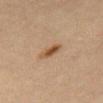No biopsy was performed on this lesion — it was imaged during a full skin examination and was not determined to be concerning. The tile uses cross-polarized illumination. On the abdomen. Automated image analysis of the tile measured a footprint of about 3.5 mm², an eccentricity of roughly 0.9, and a symmetry-axis asymmetry near 0.25. It also reported an automated nevus-likeness rating near 95 out of 100 and a lesion-detection confidence of about 100/100. A close-up tile cropped from a whole-body skin photograph, about 15 mm across. About 3 mm across. The patient is a female about 65 years old.